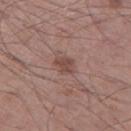{
  "biopsy_status": "not biopsied; imaged during a skin examination",
  "site": "left thigh",
  "patient": {
    "sex": "male",
    "age_approx": 60
  },
  "image": {
    "source": "total-body photography crop",
    "field_of_view_mm": 15
  }
}